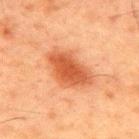Imaged during a routine full-body skin examination; the lesion was not biopsied and no histopathology is available. Longest diameter approximately 6 mm. The patient is a male approximately 60 years of age. The tile uses cross-polarized illumination. On the upper back. Cropped from a total-body skin-imaging series; the visible field is about 15 mm.